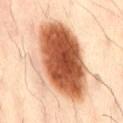biopsy status = catalogued during a skin exam; not biopsied
image source = ~15 mm crop, total-body skin-cancer survey
subject = male, about 40 years old
body site = the lower back